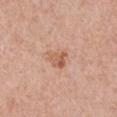workup: no biopsy performed (imaged during a skin exam); tile lighting: white-light; anatomic site: the left upper arm; diameter: ~2.5 mm (longest diameter); image source: ~15 mm crop, total-body skin-cancer survey; patient: male, aged around 70.